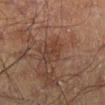No biopsy was performed on this lesion — it was imaged during a full skin examination and was not determined to be concerning. A lesion tile, about 15 mm wide, cut from a 3D total-body photograph. The tile uses cross-polarized illumination. Located on the right lower leg. The subject is a male in their 50s. Measured at roughly 3 mm in maximum diameter.